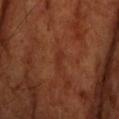This lesion was catalogued during total-body skin photography and was not selected for biopsy.
On the head or neck.
This is a cross-polarized tile.
A male patient, about 70 years old.
A 15 mm close-up extracted from a 3D total-body photography capture.